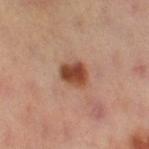<case>
  <biopsy_status>not biopsied; imaged during a skin examination</biopsy_status>
  <image>
    <source>total-body photography crop</source>
    <field_of_view_mm>15</field_of_view_mm>
  </image>
  <lighting>cross-polarized</lighting>
  <site>right thigh</site>
  <patient>
    <sex>female</sex>
    <age_approx>40</age_approx>
  </patient>
</case>